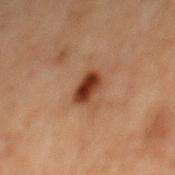Assessment: The lesion was tiled from a total-body skin photograph and was not biopsied. Background: From the mid back. A 15 mm close-up tile from a total-body photography series done for melanoma screening. A male patient aged around 70.